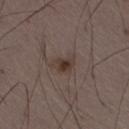<record>
  <biopsy_status>not biopsied; imaged during a skin examination</biopsy_status>
  <patient>
    <sex>male</sex>
    <age_approx>50</age_approx>
  </patient>
  <image>
    <source>total-body photography crop</source>
    <field_of_view_mm>15</field_of_view_mm>
  </image>
  <site>right thigh</site>
</record>